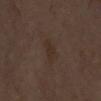biopsy status=total-body-photography surveillance lesion; no biopsy
lesion diameter=about 2.5 mm
image-analysis metrics=two-axis asymmetry of about 0.35; an average lesion color of about L≈29 a*≈13 b*≈21 (CIELAB), about 4 CIELAB-L* units darker than the surrounding skin, and a normalized border contrast of about 5.5; a nevus-likeness score of about 0/100 and a lesion-detection confidence of about 100/100
anatomic site=the right forearm
illumination=cross-polarized illumination
acquisition=~15 mm tile from a whole-body skin photo
patient=female, aged around 55